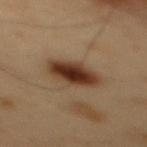No biopsy was performed on this lesion — it was imaged during a full skin examination and was not determined to be concerning. A roughly 15 mm field-of-view crop from a total-body skin photograph. The patient is a male approximately 55 years of age. On the mid back.